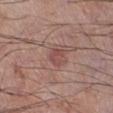<lesion>
<biopsy_status>not biopsied; imaged during a skin examination</biopsy_status>
<site>right lower leg</site>
<lesion_size>
  <long_diameter_mm_approx>2.5</long_diameter_mm_approx>
</lesion_size>
<patient>
  <sex>male</sex>
  <age_approx>70</age_approx>
</patient>
<lighting>white-light</lighting>
<image>
  <source>total-body photography crop</source>
  <field_of_view_mm>15</field_of_view_mm>
</image>
</lesion>